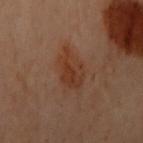  biopsy_status: not biopsied; imaged during a skin examination
  image:
    source: total-body photography crop
    field_of_view_mm: 15
  site: left arm
  lighting: cross-polarized
  patient:
    sex: female
    age_approx: 60
  automated_metrics:
    area_mm2_approx: 11.0
    eccentricity: 0.75
    shape_asymmetry: 0.25
    cielab_L: 31
    cielab_a: 19
    cielab_b: 26
    vs_skin_darker_L: 6.0
    vs_skin_contrast_norm: 7.0
  lesion_size:
    long_diameter_mm_approx: 4.5